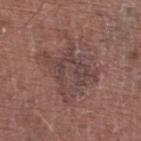biopsy_status: not biopsied; imaged during a skin examination
automated_metrics:
  border_irregularity_0_10: 7.5
  peripheral_color_asymmetry: 1.5
  nevus_likeness_0_100: 0
lesion_size:
  long_diameter_mm_approx: 7.5
patient:
  sex: male
  age_approx: 75
image:
  source: total-body photography crop
  field_of_view_mm: 15
lighting: white-light
site: leg